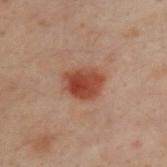biopsy status: total-body-photography surveillance lesion; no biopsy | anatomic site: the upper back | image source: 15 mm crop, total-body photography | patient: male, aged 28 to 32 | size: ≈4 mm.